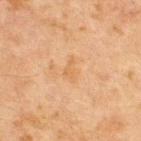{"biopsy_status": "not biopsied; imaged during a skin examination", "lighting": "cross-polarized", "image": {"source": "total-body photography crop", "field_of_view_mm": 15}, "patient": {"sex": "male", "age_approx": 65}, "lesion_size": {"long_diameter_mm_approx": 3.0}, "site": "chest"}